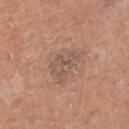workup: no biopsy performed (imaged during a skin exam); body site: the right lower leg; patient: male, aged approximately 75; acquisition: ~15 mm tile from a whole-body skin photo.